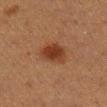{"biopsy_status": "not biopsied; imaged during a skin examination", "automated_metrics": {"area_mm2_approx": 8.0, "eccentricity": 0.6, "shape_asymmetry": 0.2, "vs_skin_darker_L": 9.0, "vs_skin_contrast_norm": 9.0, "border_irregularity_0_10": 2.0, "color_variation_0_10": 3.0, "peripheral_color_asymmetry": 1.0}, "site": "right lower leg", "image": {"source": "total-body photography crop", "field_of_view_mm": 15}, "patient": {"sex": "female", "age_approx": 40}}